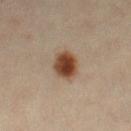Assessment:
No biopsy was performed on this lesion — it was imaged during a full skin examination and was not determined to be concerning.
Clinical summary:
The lesion is on the right lower leg. Imaged with cross-polarized lighting. An algorithmic analysis of the crop reported an area of roughly 8 mm², a shape eccentricity near 0.75, and two-axis asymmetry of about 0.15. The analysis additionally found a normalized border contrast of about 12.5. And it measured internal color variation of about 4.5 on a 0–10 scale and radial color variation of about 1.5. It also reported a nevus-likeness score of about 100/100 and a detector confidence of about 100 out of 100 that the crop contains a lesion. Cropped from a total-body skin-imaging series; the visible field is about 15 mm. A female patient, approximately 45 years of age.